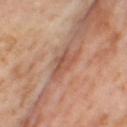Q: Is there a histopathology result?
A: catalogued during a skin exam; not biopsied